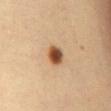Case summary:
* follow-up · imaged on a skin check; not biopsied
* image · ~15 mm tile from a whole-body skin photo
* site · the chest
* patient · female, approximately 45 years of age
* illumination · cross-polarized
* lesion size · ≈2.5 mm